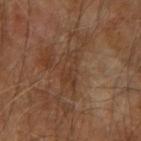Impression: This lesion was catalogued during total-body skin photography and was not selected for biopsy. Image and clinical context: This is a cross-polarized tile. The total-body-photography lesion software estimated a lesion area of about 6 mm², a shape eccentricity near 0.9, and two-axis asymmetry of about 0.35. And it measured a classifier nevus-likeness of about 0/100 and a lesion-detection confidence of about 80/100. A 15 mm close-up tile from a total-body photography series done for melanoma screening. Measured at roughly 4.5 mm in maximum diameter. On the arm. A male subject, roughly 70 years of age.